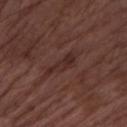No biopsy was performed on this lesion — it was imaged during a full skin examination and was not determined to be concerning. Approximately 4.5 mm at its widest. The lesion is located on the left forearm. A lesion tile, about 15 mm wide, cut from a 3D total-body photograph. A male subject, aged around 75. The lesion-visualizer software estimated a lesion color around L≈28 a*≈19 b*≈20 in CIELAB, about 6 CIELAB-L* units darker than the surrounding skin, and a normalized border contrast of about 6.5. And it measured an automated nevus-likeness rating near 0 out of 100 and a detector confidence of about 60 out of 100 that the crop contains a lesion. The tile uses white-light illumination.